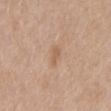Part of a total-body skin-imaging series; this lesion was reviewed on a skin check and was not flagged for biopsy. A female subject aged around 60. The total-body-photography lesion software estimated a shape eccentricity near 0.9 and a shape-asymmetry score of about 0.35 (0 = symmetric). A close-up tile cropped from a whole-body skin photograph, about 15 mm across. The lesion is on the mid back. Captured under white-light illumination. About 3 mm across.